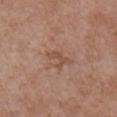This lesion was catalogued during total-body skin photography and was not selected for biopsy.
From the chest.
The subject is a female aged approximately 75.
Cropped from a total-body skin-imaging series; the visible field is about 15 mm.